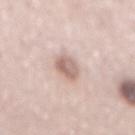<tbp_lesion>
  <biopsy_status>not biopsied; imaged during a skin examination</biopsy_status>
  <lesion_size>
    <long_diameter_mm_approx>4.0</long_diameter_mm_approx>
  </lesion_size>
  <image>
    <source>total-body photography crop</source>
    <field_of_view_mm>15</field_of_view_mm>
  </image>
  <site>mid back</site>
  <patient>
    <sex>female</sex>
    <age_approx>50</age_approx>
  </patient>
</tbp_lesion>